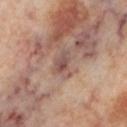follow-up = total-body-photography surveillance lesion; no biopsy | size = ~3 mm (longest diameter) | subject = female, in their mid- to late 50s | image = total-body-photography crop, ~15 mm field of view | illumination = cross-polarized illumination | site = the left lower leg.